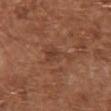workup: total-body-photography surveillance lesion; no biopsy
location: the chest
automated metrics: a lesion color around L≈41 a*≈22 b*≈29 in CIELAB; lesion-presence confidence of about 100/100
acquisition: 15 mm crop, total-body photography
subject: female, in their mid-70s
illumination: white-light